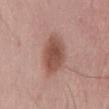This lesion was catalogued during total-body skin photography and was not selected for biopsy. The recorded lesion diameter is about 5.5 mm. A male patient about 55 years old. The lesion-visualizer software estimated an area of roughly 13 mm², a shape eccentricity near 0.8, and a shape-asymmetry score of about 0.15 (0 = symmetric). And it measured an average lesion color of about L≈51 a*≈22 b*≈26 (CIELAB) and about 12 CIELAB-L* units darker than the surrounding skin. And it measured border irregularity of about 1.5 on a 0–10 scale, internal color variation of about 3.5 on a 0–10 scale, and a peripheral color-asymmetry measure near 1. It also reported a nevus-likeness score of about 90/100 and a detector confidence of about 100 out of 100 that the crop contains a lesion. Located on the lower back. A roughly 15 mm field-of-view crop from a total-body skin photograph. Imaged with white-light lighting.